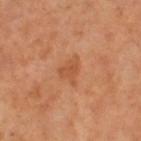Clinical impression: Recorded during total-body skin imaging; not selected for excision or biopsy. Acquisition and patient details: Measured at roughly 3.5 mm in maximum diameter. The lesion is located on the left thigh. Cropped from a total-body skin-imaging series; the visible field is about 15 mm. The patient is a female about 55 years old. This is a cross-polarized tile.